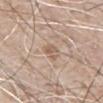| field | value |
|---|---|
| biopsy status | catalogued during a skin exam; not biopsied |
| automated lesion analysis | about 8 CIELAB-L* units darker than the surrounding skin and a normalized border contrast of about 6; border irregularity of about 4.5 on a 0–10 scale and a peripheral color-asymmetry measure near 0.5; a classifier nevus-likeness of about 0/100 and a detector confidence of about 100 out of 100 that the crop contains a lesion |
| subject | male, aged 78 to 82 |
| lesion size | ≈2.5 mm |
| image source | total-body-photography crop, ~15 mm field of view |
| site | the abdomen |